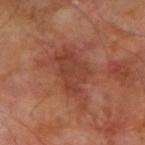{
  "biopsy_status": "not biopsied; imaged during a skin examination"
}